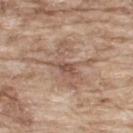Assessment:
Part of a total-body skin-imaging series; this lesion was reviewed on a skin check and was not flagged for biopsy.
Acquisition and patient details:
The tile uses white-light illumination. Located on the upper back. Measured at roughly 5.5 mm in maximum diameter. The patient is a male about 65 years old. A 15 mm close-up extracted from a 3D total-body photography capture.